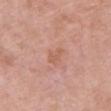biopsy status = no biopsy performed (imaged during a skin exam) | anatomic site = the chest | subject = female, approximately 70 years of age | image = ~15 mm crop, total-body skin-cancer survey.